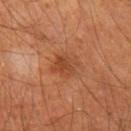Imaged during a routine full-body skin examination; the lesion was not biopsied and no histopathology is available.
The recorded lesion diameter is about 3.5 mm.
On the leg.
Automated image analysis of the tile measured a shape eccentricity near 0.65 and a shape-asymmetry score of about 0.2 (0 = symmetric). And it measured a lesion–skin lightness drop of about 7 and a lesion-to-skin contrast of about 6 (normalized; higher = more distinct). And it measured a border-irregularity rating of about 2.5/10. It also reported a classifier nevus-likeness of about 30/100 and a detector confidence of about 100 out of 100 that the crop contains a lesion.
A roughly 15 mm field-of-view crop from a total-body skin photograph.
Captured under cross-polarized illumination.
The patient is a male approximately 65 years of age.